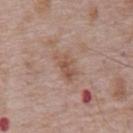Q: Is there a histopathology result?
A: total-body-photography surveillance lesion; no biopsy
Q: Where on the body is the lesion?
A: the abdomen
Q: What are the patient's age and sex?
A: male, aged 68 to 72
Q: How was this image acquired?
A: 15 mm crop, total-body photography